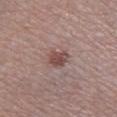The lesion was photographed on a routine skin check and not biopsied; there is no pathology result. Cropped from a whole-body photographic skin survey; the tile spans about 15 mm. A male subject, in their mid-50s. From the leg.